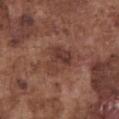Imaged during a routine full-body skin examination; the lesion was not biopsied and no histopathology is available.
About 4.5 mm across.
The lesion is on the chest.
Cropped from a whole-body photographic skin survey; the tile spans about 15 mm.
A male subject aged 73 to 77.
Automated image analysis of the tile measured a footprint of about 10 mm², an outline eccentricity of about 0.65 (0 = round, 1 = elongated), and a symmetry-axis asymmetry near 0.4. The software also gave a nevus-likeness score of about 10/100 and a detector confidence of about 100 out of 100 that the crop contains a lesion.
Captured under white-light illumination.